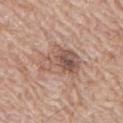The lesion was photographed on a routine skin check and not biopsied; there is no pathology result.
The lesion is located on the right upper arm.
The subject is a male aged approximately 70.
The total-body-photography lesion software estimated a lesion area of about 15 mm², an outline eccentricity of about 0.65 (0 = round, 1 = elongated), and a shape-asymmetry score of about 0.3 (0 = symmetric). The software also gave an average lesion color of about L≈55 a*≈19 b*≈26 (CIELAB), a lesion–skin lightness drop of about 10, and a normalized lesion–skin contrast near 6.5. The analysis additionally found a border-irregularity index near 3.5/10, internal color variation of about 8.5 on a 0–10 scale, and a peripheral color-asymmetry measure near 3.
Captured under white-light illumination.
A region of skin cropped from a whole-body photographic capture, roughly 15 mm wide.